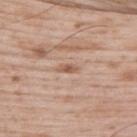Assessment: Imaged during a routine full-body skin examination; the lesion was not biopsied and no histopathology is available. Context: Automated image analysis of the tile measured a footprint of about 2.5 mm², an eccentricity of roughly 0.9, and a shape-asymmetry score of about 0.2 (0 = symmetric). The analysis additionally found a lesion color around L≈57 a*≈19 b*≈30 in CIELAB, about 9 CIELAB-L* units darker than the surrounding skin, and a normalized border contrast of about 7. On the back. This image is a 15 mm lesion crop taken from a total-body photograph. A male subject, in their 50s.